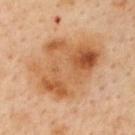Recorded during total-body skin imaging; not selected for excision or biopsy. Imaged with cross-polarized lighting. The lesion is located on the upper back. A region of skin cropped from a whole-body photographic capture, roughly 15 mm wide. The subject is a female aged approximately 45.